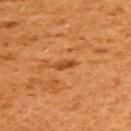Imaged during a routine full-body skin examination; the lesion was not biopsied and no histopathology is available. From the upper back. A region of skin cropped from a whole-body photographic capture, roughly 15 mm wide. Automated image analysis of the tile measured an area of roughly 2.5 mm² and two-axis asymmetry of about 0.45. It also reported a mean CIELAB color near L≈51 a*≈32 b*≈47 and a lesion-to-skin contrast of about 8 (normalized; higher = more distinct). It also reported a nevus-likeness score of about 15/100. A female patient aged around 55. Imaged with cross-polarized lighting. The recorded lesion diameter is about 2.5 mm.